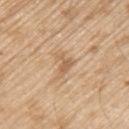{"biopsy_status": "not biopsied; imaged during a skin examination", "patient": {"sex": "male", "age_approx": 70}, "lesion_size": {"long_diameter_mm_approx": 3.0}, "image": {"source": "total-body photography crop", "field_of_view_mm": 15}, "site": "left upper arm", "lighting": "white-light", "automated_metrics": {"color_variation_0_10": 0.5, "peripheral_color_asymmetry": 0.0}}